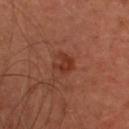Recorded during total-body skin imaging; not selected for excision or biopsy.
Cropped from a total-body skin-imaging series; the visible field is about 15 mm.
Approximately 2.5 mm at its widest.
The lesion is located on the head or neck.
A female subject roughly 45 years of age.